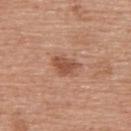The lesion was photographed on a routine skin check and not biopsied; there is no pathology result. The lesion is located on the upper back. A roughly 15 mm field-of-view crop from a total-body skin photograph. This is a white-light tile. The total-body-photography lesion software estimated an average lesion color of about L≈51 a*≈23 b*≈31 (CIELAB), roughly 11 lightness units darker than nearby skin, and a lesion-to-skin contrast of about 7.5 (normalized; higher = more distinct). Longest diameter approximately 3.5 mm. The subject is a female approximately 60 years of age.